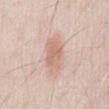Clinical impression:
Captured during whole-body skin photography for melanoma surveillance; the lesion was not biopsied.
Background:
The lesion-visualizer software estimated a footprint of about 9.5 mm² and two-axis asymmetry of about 0.25. The lesion is located on the abdomen. Approximately 4 mm at its widest. A male subject in their mid-20s. A lesion tile, about 15 mm wide, cut from a 3D total-body photograph. The tile uses white-light illumination.